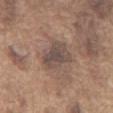notes = no biopsy performed (imaged during a skin exam)
anatomic site = the chest
lighting = white-light
lesion diameter = ≈4.5 mm
image source = 15 mm crop, total-body photography
subject = male, aged 73 to 77
automated lesion analysis = a border-irregularity rating of about 3.5/10, a within-lesion color-variation index near 3/10, and a peripheral color-asymmetry measure near 1